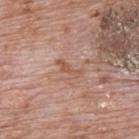Recorded during total-body skin imaging; not selected for excision or biopsy.
A male patient approximately 70 years of age.
From the upper back.
A 15 mm crop from a total-body photograph taken for skin-cancer surveillance.
This is a white-light tile.